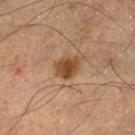Captured during whole-body skin photography for melanoma surveillance; the lesion was not biopsied. This is a cross-polarized tile. A male patient, aged 53–57. Cropped from a total-body skin-imaging series; the visible field is about 15 mm. Located on the leg. The recorded lesion diameter is about 3 mm.